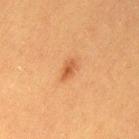Captured during whole-body skin photography for melanoma surveillance; the lesion was not biopsied.
The lesion is on the left thigh.
The tile uses cross-polarized illumination.
Automated image analysis of the tile measured a lesion area of about 2.5 mm², an outline eccentricity of about 0.85 (0 = round, 1 = elongated), and two-axis asymmetry of about 0.2. And it measured a mean CIELAB color near L≈46 a*≈24 b*≈36 and roughly 9 lightness units darker than nearby skin. The analysis additionally found a classifier nevus-likeness of about 80/100 and lesion-presence confidence of about 100/100.
Longest diameter approximately 2.5 mm.
A close-up tile cropped from a whole-body skin photograph, about 15 mm across.
A female patient aged 38 to 42.